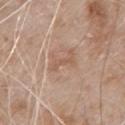notes: catalogued during a skin exam; not biopsied | image-analysis metrics: a lesion area of about 2.5 mm², an eccentricity of roughly 0.95, and a shape-asymmetry score of about 0.5 (0 = symmetric); a mean CIELAB color near L≈56 a*≈19 b*≈29 and a lesion-to-skin contrast of about 5 (normalized; higher = more distinct) | lesion diameter: ≈3 mm | body site: the chest | lighting: white-light | patient: male, aged approximately 80 | acquisition: ~15 mm crop, total-body skin-cancer survey.